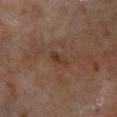The lesion was photographed on a routine skin check and not biopsied; there is no pathology result. Imaged with cross-polarized lighting. From the right lower leg. The lesion-visualizer software estimated an outline eccentricity of about 0.85 (0 = round, 1 = elongated). The software also gave a classifier nevus-likeness of about 5/100 and a detector confidence of about 100 out of 100 that the crop contains a lesion. About 2.5 mm across. The subject is a male aged approximately 60. A close-up tile cropped from a whole-body skin photograph, about 15 mm across.